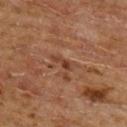workup = total-body-photography surveillance lesion; no biopsy
tile lighting = cross-polarized illumination
size = ≈3 mm
patient = male, aged 63–67
image = total-body-photography crop, ~15 mm field of view
body site = the upper back